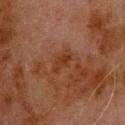| key | value |
|---|---|
| image source | ~15 mm tile from a whole-body skin photo |
| body site | the upper back |
| patient | male, in their 80s |
| lesion diameter | about 3 mm |
| tile lighting | cross-polarized illumination |
| automated lesion analysis | about 5 CIELAB-L* units darker than the surrounding skin and a lesion-to-skin contrast of about 7 (normalized; higher = more distinct); border irregularity of about 3.5 on a 0–10 scale, a within-lesion color-variation index near 2/10, and peripheral color asymmetry of about 1; an automated nevus-likeness rating near 0 out of 100 and lesion-presence confidence of about 100/100 |